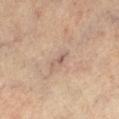Q: Was a biopsy performed?
A: catalogued during a skin exam; not biopsied
Q: Patient demographics?
A: female, roughly 65 years of age
Q: Illumination type?
A: cross-polarized
Q: Lesion location?
A: the left lower leg
Q: How large is the lesion?
A: about 3 mm
Q: What is the imaging modality?
A: 15 mm crop, total-body photography